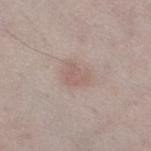Case summary:
* notes · no biopsy performed (imaged during a skin exam)
* size · about 3 mm
* subject · female, roughly 40 years of age
* location · the right thigh
* automated lesion analysis · internal color variation of about 1.5 on a 0–10 scale and peripheral color asymmetry of about 0.5; a lesion-detection confidence of about 100/100
* tile lighting · white-light
* acquisition · ~15 mm tile from a whole-body skin photo